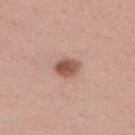{"patient": {"sex": "male", "age_approx": 40}, "site": "back", "image": {"source": "total-body photography crop", "field_of_view_mm": 15}}